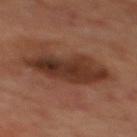site: the mid back
image-analysis metrics: an eccentricity of roughly 0.85 and two-axis asymmetry of about 0.2; a lesion color around L≈32 a*≈20 b*≈26 in CIELAB
acquisition: ~15 mm crop, total-body skin-cancer survey
patient: male, approximately 70 years of age